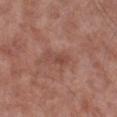The lesion was tiled from a total-body skin photograph and was not biopsied.